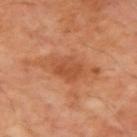Recorded during total-body skin imaging; not selected for excision or biopsy. A male patient aged around 70. A region of skin cropped from a whole-body photographic capture, roughly 15 mm wide. On the right upper arm. This is a cross-polarized tile.